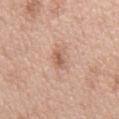| key | value |
|---|---|
| notes | total-body-photography surveillance lesion; no biopsy |
| subject | male, aged approximately 50 |
| image | ~15 mm crop, total-body skin-cancer survey |
| body site | the chest |